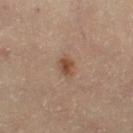{
  "biopsy_status": "not biopsied; imaged during a skin examination",
  "image": {
    "source": "total-body photography crop",
    "field_of_view_mm": 15
  },
  "lighting": "cross-polarized",
  "patient": {
    "sex": "female",
    "age_approx": 45
  },
  "site": "left thigh",
  "automated_metrics": {
    "cielab_L": 47,
    "cielab_a": 19,
    "cielab_b": 29,
    "vs_skin_darker_L": 9.0,
    "vs_skin_contrast_norm": 8.0,
    "border_irregularity_0_10": 2.0,
    "color_variation_0_10": 3.5
  },
  "lesion_size": {
    "long_diameter_mm_approx": 2.5
  }
}